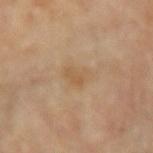{"biopsy_status": "not biopsied; imaged during a skin examination", "lesion_size": {"long_diameter_mm_approx": 3.0}, "patient": {"sex": "female", "age_approx": 65}, "site": "arm", "lighting": "cross-polarized", "image": {"source": "total-body photography crop", "field_of_view_mm": 15}, "automated_metrics": {"cielab_L": 58, "cielab_a": 16, "cielab_b": 36, "vs_skin_darker_L": 6.0, "vs_skin_contrast_norm": 5.0, "border_irregularity_0_10": 3.0, "color_variation_0_10": 2.0, "peripheral_color_asymmetry": 0.5, "lesion_detection_confidence_0_100": 100}}